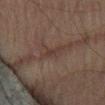Impression:
The lesion was photographed on a routine skin check and not biopsied; there is no pathology result.
Image and clinical context:
A 15 mm crop from a total-body photograph taken for skin-cancer surveillance. On the right thigh. A male patient, aged around 75.